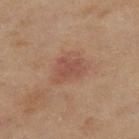Clinical impression:
Recorded during total-body skin imaging; not selected for excision or biopsy.
Context:
Longest diameter approximately 4 mm. This image is a 15 mm lesion crop taken from a total-body photograph. A female subject, aged 58–62. The lesion is on the right thigh.